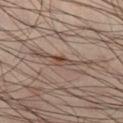Findings:
* biopsy status · imaged on a skin check; not biopsied